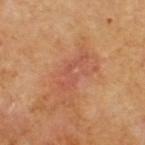Clinical impression: No biopsy was performed on this lesion — it was imaged during a full skin examination and was not determined to be concerning. Acquisition and patient details: A close-up tile cropped from a whole-body skin photograph, about 15 mm across. Automated tile analysis of the lesion measured a mean CIELAB color near L≈51 a*≈26 b*≈32, about 6 CIELAB-L* units darker than the surrounding skin, and a normalized lesion–skin contrast near 5. The software also gave a border-irregularity index near 9.5/10, a within-lesion color-variation index near 0/10, and a peripheral color-asymmetry measure near 0. It also reported a nevus-likeness score of about 0/100 and lesion-presence confidence of about 100/100. On the head or neck. A male subject aged approximately 85 or older. Captured under cross-polarized illumination. The recorded lesion diameter is about 5 mm.